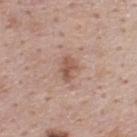Notes:
* biopsy status — no biopsy performed (imaged during a skin exam)
* TBP lesion metrics — a lesion area of about 5 mm² and a shape-asymmetry score of about 0.25 (0 = symmetric); a within-lesion color-variation index near 2.5/10 and a peripheral color-asymmetry measure near 1; a nevus-likeness score of about 30/100 and lesion-presence confidence of about 100/100
* subject — male, aged approximately 45
* location — the upper back
* lighting — white-light
* image — ~15 mm tile from a whole-body skin photo
* diameter — ~3 mm (longest diameter)